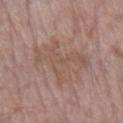{"biopsy_status": "not biopsied; imaged during a skin examination", "patient": {"sex": "female", "age_approx": 65}, "lesion_size": {"long_diameter_mm_approx": 6.0}, "automated_metrics": {"eccentricity": 0.65, "shape_asymmetry": 0.5, "cielab_L": 53, "cielab_a": 17, "cielab_b": 25, "vs_skin_darker_L": 5.0, "vs_skin_contrast_norm": 4.5, "border_irregularity_0_10": 9.0, "color_variation_0_10": 2.5, "peripheral_color_asymmetry": 1.0, "nevus_likeness_0_100": 0}, "lighting": "white-light", "image": {"source": "total-body photography crop", "field_of_view_mm": 15}, "site": "left forearm"}